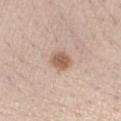Impression:
This lesion was catalogued during total-body skin photography and was not selected for biopsy.
Image and clinical context:
The lesion is located on the arm. A lesion tile, about 15 mm wide, cut from a 3D total-body photograph. Approximately 2.5 mm at its widest. The subject is a female aged approximately 40.